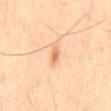notes: catalogued during a skin exam; not biopsied
image source: ~15 mm crop, total-body skin-cancer survey
patient: male, aged 58–62
tile lighting: cross-polarized illumination
automated lesion analysis: an area of roughly 2 mm² and a shape-asymmetry score of about 0.4 (0 = symmetric); an average lesion color of about L≈69 a*≈25 b*≈40 (CIELAB), roughly 11 lightness units darker than nearby skin, and a normalized border contrast of about 7
diameter: about 2 mm
location: the back